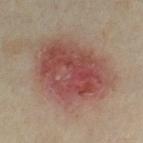notes=total-body-photography surveillance lesion; no biopsy
location=the leg
patient=female, aged 33–37
imaging modality=total-body-photography crop, ~15 mm field of view